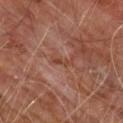biopsy_status: not biopsied; imaged during a skin examination
image:
  source: total-body photography crop
  field_of_view_mm: 15
lesion_size:
  long_diameter_mm_approx: 2.5
site: chest
patient:
  sex: male
  age_approx: 60